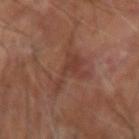Clinical impression:
The lesion was photographed on a routine skin check and not biopsied; there is no pathology result.
Background:
The lesion is located on the right forearm. The patient is a male aged 68–72. Cropped from a whole-body photographic skin survey; the tile spans about 15 mm. Imaged with cross-polarized lighting. Approximately 6.5 mm at its widest.